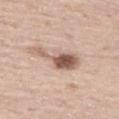Q: Is there a histopathology result?
A: total-body-photography surveillance lesion; no biopsy